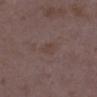{"biopsy_status": "not biopsied; imaged during a skin examination", "lesion_size": {"long_diameter_mm_approx": 2.5}, "site": "left lower leg", "image": {"source": "total-body photography crop", "field_of_view_mm": 15}, "automated_metrics": {"cielab_L": 41, "cielab_a": 15, "cielab_b": 20, "vs_skin_darker_L": 4.0, "vs_skin_contrast_norm": 5.0, "lesion_detection_confidence_0_100": 100}, "patient": {"sex": "female", "age_approx": 35}}